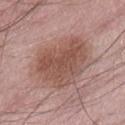Recorded during total-body skin imaging; not selected for excision or biopsy. Cropped from a whole-body photographic skin survey; the tile spans about 15 mm. Imaged with white-light lighting. A male patient in their mid-60s. Measured at roughly 8 mm in maximum diameter. From the abdomen. The lesion-visualizer software estimated a border-irregularity index near 2/10 and a within-lesion color-variation index near 4.5/10. The software also gave a classifier nevus-likeness of about 10/100 and a lesion-detection confidence of about 100/100.